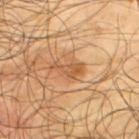Notes:
- follow-up · no biopsy performed (imaged during a skin exam)